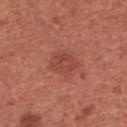site: the left forearm | acquisition: 15 mm crop, total-body photography | diameter: about 3.5 mm | subject: female, in their 50s | TBP lesion metrics: a mean CIELAB color near L≈46 a*≈29 b*≈29, roughly 7 lightness units darker than nearby skin, and a normalized lesion–skin contrast near 5.5; a border-irregularity index near 2/10, a color-variation rating of about 2.5/10, and peripheral color asymmetry of about 1; a classifier nevus-likeness of about 30/100 and a lesion-detection confidence of about 100/100 | illumination: white-light illumination.